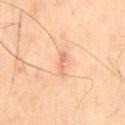<case>
<lighting>cross-polarized</lighting>
<site>back</site>
<lesion_size>
  <long_diameter_mm_approx>3.0</long_diameter_mm_approx>
</lesion_size>
<automated_metrics>
  <area_mm2_approx>2.0</area_mm2_approx>
  <eccentricity>0.95</eccentricity>
  <shape_asymmetry>0.45</shape_asymmetry>
  <vs_skin_darker_L>9.0</vs_skin_darker_L>
  <border_irregularity_0_10>5.5</border_irregularity_0_10>
  <color_variation_0_10>0.0</color_variation_0_10>
  <peripheral_color_asymmetry>0.0</peripheral_color_asymmetry>
  <nevus_likeness_0_100>0</nevus_likeness_0_100>
  <lesion_detection_confidence_0_100>100</lesion_detection_confidence_0_100>
</automated_metrics>
<patient>
  <sex>male</sex>
  <age_approx>60</age_approx>
</patient>
<image>
  <source>total-body photography crop</source>
  <field_of_view_mm>15</field_of_view_mm>
</image>
</case>